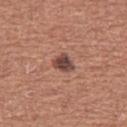The lesion was tiled from a total-body skin photograph and was not biopsied. The lesion is on the left thigh. Measured at roughly 3 mm in maximum diameter. Cropped from a total-body skin-imaging series; the visible field is about 15 mm. A female subject, in their 50s. An algorithmic analysis of the crop reported a lesion color around L≈45 a*≈21 b*≈23 in CIELAB, roughly 14 lightness units darker than nearby skin, and a normalized border contrast of about 10.5. It also reported a border-irregularity index near 2.5/10 and a within-lesion color-variation index near 4/10. The analysis additionally found an automated nevus-likeness rating near 70 out of 100 and a detector confidence of about 100 out of 100 that the crop contains a lesion. Captured under white-light illumination.